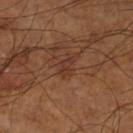The tile uses cross-polarized illumination. Automated tile analysis of the lesion measured a lesion area of about 2.5 mm², an outline eccentricity of about 0.85 (0 = round, 1 = elongated), and two-axis asymmetry of about 0.4. The software also gave an average lesion color of about L≈33 a*≈21 b*≈26 (CIELAB), a lesion–skin lightness drop of about 6, and a normalized border contrast of about 6. On the left lower leg. Approximately 2.5 mm at its widest. A region of skin cropped from a whole-body photographic capture, roughly 15 mm wide. A male subject, about 60 years old.